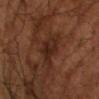{
  "patient": {
    "sex": "male",
    "age_approx": 65
  },
  "image": {
    "source": "total-body photography crop",
    "field_of_view_mm": 15
  }
}